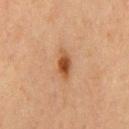follow-up: no biopsy performed (imaged during a skin exam)
lighting: cross-polarized
anatomic site: the chest
image: total-body-photography crop, ~15 mm field of view
lesion size: ~3.5 mm (longest diameter)
subject: male, in their mid- to late 60s
image-analysis metrics: an area of roughly 4.5 mm², an eccentricity of roughly 0.9, and a symmetry-axis asymmetry near 0.25; a mean CIELAB color near L≈43 a*≈22 b*≈34, a lesion–skin lightness drop of about 12, and a lesion-to-skin contrast of about 10 (normalized; higher = more distinct)